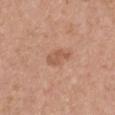biopsy_status: not biopsied; imaged during a skin examination
image:
  source: total-body photography crop
  field_of_view_mm: 15
lesion_size:
  long_diameter_mm_approx: 3.5
site: chest
patient:
  sex: female
  age_approx: 40